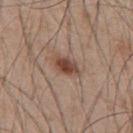Clinical impression:
This lesion was catalogued during total-body skin photography and was not selected for biopsy.
Clinical summary:
The patient is a male in their 50s. A close-up tile cropped from a whole-body skin photograph, about 15 mm across. An algorithmic analysis of the crop reported a lesion color around L≈45 a*≈20 b*≈26 in CIELAB, about 12 CIELAB-L* units darker than the surrounding skin, and a lesion-to-skin contrast of about 9.5 (normalized; higher = more distinct). Imaged with white-light lighting. About 3.5 mm across. From the upper back.